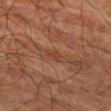Q: Is there a histopathology result?
A: imaged on a skin check; not biopsied
Q: Who is the patient?
A: male, aged 78–82
Q: What is the imaging modality?
A: total-body-photography crop, ~15 mm field of view
Q: Illumination type?
A: cross-polarized
Q: Lesion location?
A: the left thigh
Q: What did automated image analysis measure?
A: border irregularity of about 3 on a 0–10 scale, a color-variation rating of about 1.5/10, and a peripheral color-asymmetry measure near 0.5
Q: How large is the lesion?
A: ~3 mm (longest diameter)